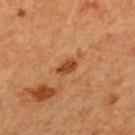No biopsy was performed on this lesion — it was imaged during a full skin examination and was not determined to be concerning.
On the upper back.
The tile uses cross-polarized illumination.
The lesion's longest dimension is about 2.5 mm.
A male patient, roughly 65 years of age.
A 15 mm close-up extracted from a 3D total-body photography capture.